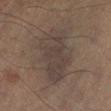Imaged during a routine full-body skin examination; the lesion was not biopsied and no histopathology is available.